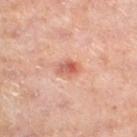Imaged during a routine full-body skin examination; the lesion was not biopsied and no histopathology is available. A male patient, aged around 65. Approximately 3 mm at its widest. This image is a 15 mm lesion crop taken from a total-body photograph. Automated image analysis of the tile measured a lesion area of about 4.5 mm² and a shape-asymmetry score of about 0.25 (0 = symmetric). This is a cross-polarized tile. Located on the left lower leg.